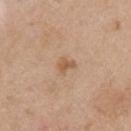follow-up: imaged on a skin check; not biopsied
location: the right upper arm
acquisition: ~15 mm crop, total-body skin-cancer survey
patient: male, aged 53 to 57
image-analysis metrics: a lesion color around L≈57 a*≈19 b*≈34 in CIELAB, about 9 CIELAB-L* units darker than the surrounding skin, and a lesion-to-skin contrast of about 7 (normalized; higher = more distinct)
tile lighting: white-light
lesion size: ≈2 mm